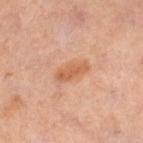Clinical impression:
Recorded during total-body skin imaging; not selected for excision or biopsy.
Clinical summary:
The patient is a female approximately 50 years of age. This image is a 15 mm lesion crop taken from a total-body photograph. The lesion is on the right thigh.